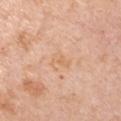Q: Was a biopsy performed?
A: imaged on a skin check; not biopsied